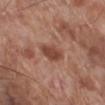Imaged during a routine full-body skin examination; the lesion was not biopsied and no histopathology is available. The tile uses white-light illumination. Longest diameter approximately 3.5 mm. From the right lower leg. A roughly 15 mm field-of-view crop from a total-body skin photograph. A male subject aged approximately 70.